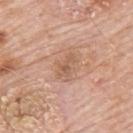  biopsy_status: not biopsied; imaged during a skin examination
  site: upper back
  automated_metrics:
    area_mm2_approx: 3.0
    eccentricity: 0.9
    shape_asymmetry: 0.45
    cielab_L: 57
    cielab_a: 21
    cielab_b: 31
    vs_skin_darker_L: 8.0
    vs_skin_contrast_norm: 5.5
    border_irregularity_0_10: 5.0
    color_variation_0_10: 0.5
    peripheral_color_asymmetry: 0.0
    nevus_likeness_0_100: 0
  lighting: white-light
  image:
    source: total-body photography crop
    field_of_view_mm: 15
  patient:
    sex: male
    age_approx: 80